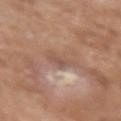Clinical impression:
This lesion was catalogued during total-body skin photography and was not selected for biopsy.
Background:
A male subject, about 65 years old. Cropped from a total-body skin-imaging series; the visible field is about 15 mm. About 3.5 mm across. Automated tile analysis of the lesion measured an area of roughly 5 mm² and two-axis asymmetry of about 0.45. From the left upper arm. This is a white-light tile.